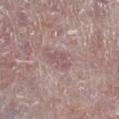| field | value |
|---|---|
| notes | total-body-photography surveillance lesion; no biopsy |
| patient | male, approximately 75 years of age |
| imaging modality | ~15 mm crop, total-body skin-cancer survey |
| anatomic site | the right lower leg |